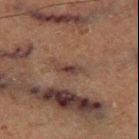The lesion was tiled from a total-body skin photograph and was not biopsied.
A roughly 15 mm field-of-view crop from a total-body skin photograph.
A male patient, in their mid-70s.
On the right thigh.